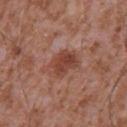No biopsy was performed on this lesion — it was imaged during a full skin examination and was not determined to be concerning. From the chest. Captured under white-light illumination. A male patient aged 43 to 47. A region of skin cropped from a whole-body photographic capture, roughly 15 mm wide. Measured at roughly 4 mm in maximum diameter.